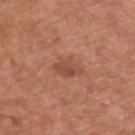Q: Is there a histopathology result?
A: total-body-photography surveillance lesion; no biopsy
Q: What are the patient's age and sex?
A: male, approximately 55 years of age
Q: What is the imaging modality?
A: ~15 mm crop, total-body skin-cancer survey
Q: What is the anatomic site?
A: the upper back